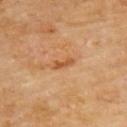anatomic site — the upper back
patient — male, about 60 years old
acquisition — ~15 mm tile from a whole-body skin photo
size — about 3 mm
automated metrics — a footprint of about 3 mm², an outline eccentricity of about 0.95 (0 = round, 1 = elongated), and a shape-asymmetry score of about 0.35 (0 = symmetric); a mean CIELAB color near L≈53 a*≈23 b*≈40; a nevus-likeness score of about 0/100 and a detector confidence of about 100 out of 100 that the crop contains a lesion